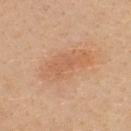This lesion was catalogued during total-body skin photography and was not selected for biopsy.